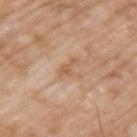Assessment: Recorded during total-body skin imaging; not selected for excision or biopsy. Acquisition and patient details: A male subject approximately 60 years of age. About 2.5 mm across. Cropped from a total-body skin-imaging series; the visible field is about 15 mm. This is a white-light tile. From the left upper arm. The total-body-photography lesion software estimated a lesion area of about 2 mm², an eccentricity of roughly 0.9, and a shape-asymmetry score of about 0.5 (0 = symmetric). The software also gave a lesion color around L≈58 a*≈20 b*≈35 in CIELAB and a normalized border contrast of about 6. The software also gave border irregularity of about 5.5 on a 0–10 scale, internal color variation of about 0 on a 0–10 scale, and radial color variation of about 0.